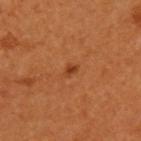Imaged during a routine full-body skin examination; the lesion was not biopsied and no histopathology is available.
On the upper back.
A 15 mm close-up extracted from a 3D total-body photography capture.
Measured at roughly 1.5 mm in maximum diameter.
A male subject aged 58 to 62.
The lesion-visualizer software estimated a lesion color around L≈38 a*≈27 b*≈37 in CIELAB, a lesion–skin lightness drop of about 9, and a normalized lesion–skin contrast near 7. The analysis additionally found a border-irregularity rating of about 3/10, a color-variation rating of about 0/10, and a peripheral color-asymmetry measure near 0. And it measured a nevus-likeness score of about 85/100 and a lesion-detection confidence of about 100/100.